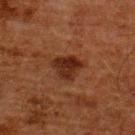patient: male, in their 60s
site: the upper back
image source: ~15 mm crop, total-body skin-cancer survey